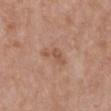biopsy_status: not biopsied; imaged during a skin examination
image:
  source: total-body photography crop
  field_of_view_mm: 15
patient:
  sex: female
  age_approx: 65
site: chest